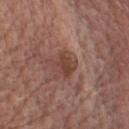Clinical summary: The lesion-visualizer software estimated a border-irregularity index near 2.5/10. And it measured a nevus-likeness score of about 30/100 and a lesion-detection confidence of about 100/100. A male patient roughly 70 years of age. The lesion's longest dimension is about 3 mm. This is a white-light tile. The lesion is located on the chest. A 15 mm crop from a total-body photograph taken for skin-cancer surveillance.